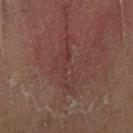Clinical impression:
The lesion was tiled from a total-body skin photograph and was not biopsied.
Background:
From the right lower leg. A male patient about 60 years old. A region of skin cropped from a whole-body photographic capture, roughly 15 mm wide.